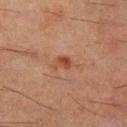Recorded during total-body skin imaging; not selected for excision or biopsy. A lesion tile, about 15 mm wide, cut from a 3D total-body photograph. The patient is a male in their 60s. The lesion is located on the right lower leg. This is a cross-polarized tile.